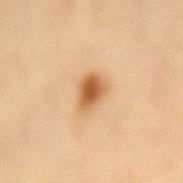{
  "biopsy_status": "not biopsied; imaged during a skin examination",
  "image": {
    "source": "total-body photography crop",
    "field_of_view_mm": 15
  },
  "lighting": "cross-polarized",
  "patient": {
    "sex": "female",
    "age_approx": 40
  },
  "lesion_size": {
    "long_diameter_mm_approx": 3.0
  },
  "site": "back"
}